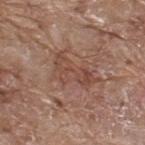Impression:
This lesion was catalogued during total-body skin photography and was not selected for biopsy.
Clinical summary:
The lesion's longest dimension is about 5.5 mm. Cropped from a whole-body photographic skin survey; the tile spans about 15 mm. From the upper back. This is a white-light tile. The subject is a male aged approximately 70.